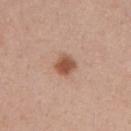Imaged during a routine full-body skin examination; the lesion was not biopsied and no histopathology is available. Imaged with white-light lighting. On the mid back. A 15 mm close-up extracted from a 3D total-body photography capture. A female patient approximately 40 years of age. Longest diameter approximately 2.5 mm. Automated tile analysis of the lesion measured border irregularity of about 1 on a 0–10 scale, a within-lesion color-variation index near 3/10, and radial color variation of about 1. And it measured a nevus-likeness score of about 100/100 and a lesion-detection confidence of about 100/100.